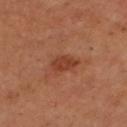Recorded during total-body skin imaging; not selected for excision or biopsy. Measured at roughly 3 mm in maximum diameter. Imaged with cross-polarized lighting. A male patient in their mid- to late 50s. Cropped from a whole-body photographic skin survey; the tile spans about 15 mm. Automated tile analysis of the lesion measured border irregularity of about 2 on a 0–10 scale and peripheral color asymmetry of about 0.5. The lesion is located on the head or neck.